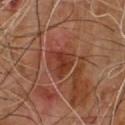follow-up: imaged on a skin check; not biopsied
patient: male, aged around 65
image: ~15 mm tile from a whole-body skin photo
lighting: cross-polarized illumination